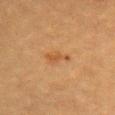A lesion tile, about 15 mm wide, cut from a 3D total-body photograph. Automated tile analysis of the lesion measured a mean CIELAB color near L≈45 a*≈21 b*≈36, about 7 CIELAB-L* units darker than the surrounding skin, and a normalized lesion–skin contrast near 6. From the chest. Imaged with cross-polarized lighting. The subject is a female roughly 55 years of age. Approximately 3 mm at its widest.